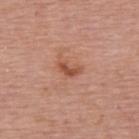Part of a total-body skin-imaging series; this lesion was reviewed on a skin check and was not flagged for biopsy.
An algorithmic analysis of the crop reported a footprint of about 3 mm², an eccentricity of roughly 0.85, and a symmetry-axis asymmetry near 0.5. And it measured a nevus-likeness score of about 15/100 and a detector confidence of about 100 out of 100 that the crop contains a lesion.
On the upper back.
The patient is a female in their mid- to late 60s.
This is a white-light tile.
A 15 mm close-up tile from a total-body photography series done for melanoma screening.